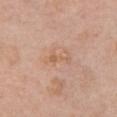Findings:
- follow-up — no biopsy performed (imaged during a skin exam)
- imaging modality — ~15 mm crop, total-body skin-cancer survey
- subject — female, aged 58 to 62
- location — the front of the torso
- size — ~3 mm (longest diameter)